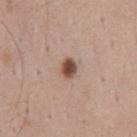Case summary:
* notes: catalogued during a skin exam; not biopsied
* TBP lesion metrics: internal color variation of about 5 on a 0–10 scale and radial color variation of about 2; an automated nevus-likeness rating near 100 out of 100 and a lesion-detection confidence of about 100/100
* subject: male, in their mid- to late 30s
* lesion size: about 2.5 mm
* image source: 15 mm crop, total-body photography
* tile lighting: white-light
* anatomic site: the mid back